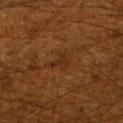| key | value |
|---|---|
| follow-up | imaged on a skin check; not biopsied |
| tile lighting | cross-polarized illumination |
| patient | male, aged 58 to 62 |
| anatomic site | the left lower leg |
| image | total-body-photography crop, ~15 mm field of view |
| TBP lesion metrics | a footprint of about 4.5 mm², an eccentricity of roughly 0.75, and a shape-asymmetry score of about 0.5 (0 = symmetric); a mean CIELAB color near L≈24 a*≈18 b*≈28 and a normalized lesion–skin contrast near 5.5 |